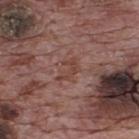biopsy_status: not biopsied; imaged during a skin examination
site: back
automated_metrics:
  cielab_L: 43
  cielab_a: 21
  cielab_b: 24
  vs_skin_darker_L: 7.0
  vs_skin_contrast_norm: 6.0
  border_irregularity_0_10: 6.0
  color_variation_0_10: 0.0
patient:
  sex: male
  age_approx: 70
lighting: white-light
image:
  source: total-body photography crop
  field_of_view_mm: 15